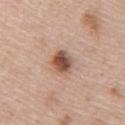Part of a total-body skin-imaging series; this lesion was reviewed on a skin check and was not flagged for biopsy.
The lesion-visualizer software estimated a footprint of about 6 mm², an outline eccentricity of about 0.4 (0 = round, 1 = elongated), and a shape-asymmetry score of about 0.2 (0 = symmetric). And it measured a mean CIELAB color near L≈51 a*≈21 b*≈28, about 16 CIELAB-L* units darker than the surrounding skin, and a lesion-to-skin contrast of about 10.5 (normalized; higher = more distinct).
A close-up tile cropped from a whole-body skin photograph, about 15 mm across.
This is a white-light tile.
The lesion is located on the right upper arm.
The patient is a female aged 48–52.